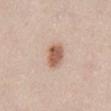biopsy_status: not biopsied; imaged during a skin examination
patient:
  sex: male
  age_approx: 40
image:
  source: total-body photography crop
  field_of_view_mm: 15
automated_metrics:
  area_mm2_approx: 6.0
  eccentricity: 0.7
  shape_asymmetry: 0.15
  nevus_likeness_0_100: 100
  lesion_detection_confidence_0_100: 100
lesion_size:
  long_diameter_mm_approx: 3.0
site: abdomen